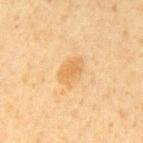Captured during whole-body skin photography for melanoma surveillance; the lesion was not biopsied. A 15 mm close-up extracted from a 3D total-body photography capture. The patient is a male in their 60s. The lesion is located on the mid back.